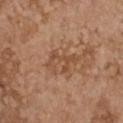Q: Is there a histopathology result?
A: catalogued during a skin exam; not biopsied
Q: What are the patient's age and sex?
A: female, in their mid- to late 60s
Q: Automated lesion metrics?
A: border irregularity of about 8.5 on a 0–10 scale, a color-variation rating of about 2.5/10, and peripheral color asymmetry of about 1
Q: How was the tile lit?
A: white-light illumination
Q: How was this image acquired?
A: ~15 mm crop, total-body skin-cancer survey
Q: What is the lesion's diameter?
A: ~4 mm (longest diameter)
Q: What is the anatomic site?
A: the chest